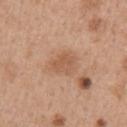This lesion was catalogued during total-body skin photography and was not selected for biopsy.
A female patient about 40 years old.
The lesion-visualizer software estimated a footprint of about 5.5 mm², an outline eccentricity of about 0.7 (0 = round, 1 = elongated), and a symmetry-axis asymmetry near 0.2. The software also gave a color-variation rating of about 2/10 and radial color variation of about 0.5. The software also gave an automated nevus-likeness rating near 0 out of 100 and a lesion-detection confidence of about 100/100.
Located on the right upper arm.
A roughly 15 mm field-of-view crop from a total-body skin photograph.
Captured under white-light illumination.
Approximately 3 mm at its widest.